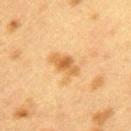Part of a total-body skin-imaging series; this lesion was reviewed on a skin check and was not flagged for biopsy.
Located on the back.
Automated image analysis of the tile measured a footprint of about 6.5 mm², a shape eccentricity near 0.75, and a shape-asymmetry score of about 0.3 (0 = symmetric). The software also gave a lesion color around L≈53 a*≈19 b*≈39 in CIELAB and a lesion–skin lightness drop of about 9.
A female patient approximately 40 years of age.
A 15 mm crop from a total-body photograph taken for skin-cancer surveillance.